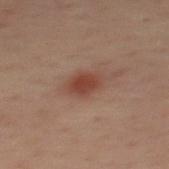biopsy status = no biopsy performed (imaged during a skin exam)
anatomic site = the mid back
subject = male, about 40 years old
imaging modality = 15 mm crop, total-body photography